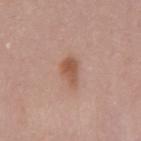Impression: Part of a total-body skin-imaging series; this lesion was reviewed on a skin check and was not flagged for biopsy. Acquisition and patient details: A close-up tile cropped from a whole-body skin photograph, about 15 mm across. About 3 mm across. The subject is a male in their mid-50s. This is a white-light tile. Located on the mid back.